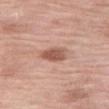Clinical impression:
No biopsy was performed on this lesion — it was imaged during a full skin examination and was not determined to be concerning.
Context:
This is a white-light tile. From the right thigh. The recorded lesion diameter is about 3.5 mm. A 15 mm close-up extracted from a 3D total-body photography capture. A female subject approximately 70 years of age.